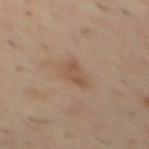Notes:
• notes: catalogued during a skin exam; not biopsied
• subject: male, approximately 55 years of age
• image: 15 mm crop, total-body photography
• anatomic site: the mid back